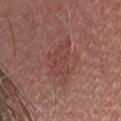Assessment: Recorded during total-body skin imaging; not selected for excision or biopsy. Image and clinical context: Longest diameter approximately 5.5 mm. Captured under white-light illumination. This image is a 15 mm lesion crop taken from a total-body photograph. The subject is a male aged 28 to 32.